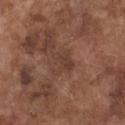biopsy_status: not biopsied; imaged during a skin examination
lighting: white-light
image:
  source: total-body photography crop
  field_of_view_mm: 15
patient:
  sex: male
  age_approx: 75
site: chest